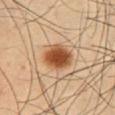location: the abdomen
image source: ~15 mm tile from a whole-body skin photo
subject: male, approximately 55 years of age
diameter: ≈4.5 mm
automated metrics: a footprint of about 10 mm², an eccentricity of roughly 0.75, and two-axis asymmetry of about 0.15; an average lesion color of about L≈49 a*≈23 b*≈36 (CIELAB), a lesion–skin lightness drop of about 16, and a lesion-to-skin contrast of about 11.5 (normalized; higher = more distinct); a classifier nevus-likeness of about 100/100 and a detector confidence of about 100 out of 100 that the crop contains a lesion
tile lighting: cross-polarized illumination